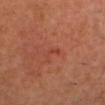Clinical impression:
Imaged during a routine full-body skin examination; the lesion was not biopsied and no histopathology is available.